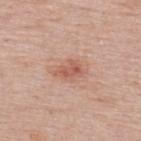notes = catalogued during a skin exam; not biopsied
location = the upper back
lesion diameter = ~3.5 mm (longest diameter)
illumination = white-light illumination
TBP lesion metrics = a footprint of about 5 mm², a shape eccentricity near 0.8, and two-axis asymmetry of about 0.35; a mean CIELAB color near L≈57 a*≈25 b*≈29 and a normalized border contrast of about 6.5; internal color variation of about 3 on a 0–10 scale and radial color variation of about 1; an automated nevus-likeness rating near 70 out of 100
image source = ~15 mm tile from a whole-body skin photo
patient = female, aged around 50